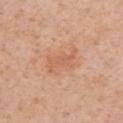Clinical impression: No biopsy was performed on this lesion — it was imaged during a full skin examination and was not determined to be concerning. Context: The lesion is located on the right upper arm. The subject is a female about 40 years old. Imaged with white-light lighting. Measured at roughly 4.5 mm in maximum diameter. A 15 mm crop from a total-body photograph taken for skin-cancer surveillance.